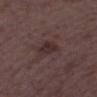{
  "biopsy_status": "not biopsied; imaged during a skin examination",
  "image": {
    "source": "total-body photography crop",
    "field_of_view_mm": 15
  },
  "automated_metrics": {
    "area_mm2_approx": 4.5,
    "shape_asymmetry": 0.3,
    "cielab_L": 28,
    "cielab_a": 16,
    "cielab_b": 16,
    "vs_skin_contrast_norm": 7.5,
    "border_irregularity_0_10": 3.0,
    "color_variation_0_10": 2.0,
    "peripheral_color_asymmetry": 0.5
  },
  "lesion_size": {
    "long_diameter_mm_approx": 3.0
  },
  "lighting": "white-light",
  "site": "right thigh",
  "patient": {
    "sex": "female",
    "age_approx": 35
  }
}